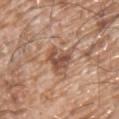follow-up = catalogued during a skin exam; not biopsied
imaging modality = ~15 mm crop, total-body skin-cancer survey
site = the chest
lesion size = ~4.5 mm (longest diameter)
patient = male, in their mid-60s